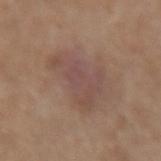The lesion was tiled from a total-body skin photograph and was not biopsied.
A close-up tile cropped from a whole-body skin photograph, about 15 mm across.
Located on the right forearm.
A male subject, aged approximately 70.
An algorithmic analysis of the crop reported a border-irregularity rating of about 6/10, a color-variation rating of about 3/10, and a peripheral color-asymmetry measure near 1.
Imaged with white-light lighting.
Longest diameter approximately 6 mm.